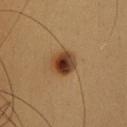biopsy status — catalogued during a skin exam; not biopsied
subject — female, in their mid-30s
body site — the head or neck
illumination — cross-polarized illumination
image — total-body-photography crop, ~15 mm field of view